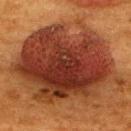No biopsy was performed on this lesion — it was imaged during a full skin examination and was not determined to be concerning.
The lesion's longest dimension is about 13 mm.
Located on the upper back.
The total-body-photography lesion software estimated a footprint of about 85 mm², a shape eccentricity near 0.65, and two-axis asymmetry of about 0.15. The analysis additionally found border irregularity of about 3.5 on a 0–10 scale and a within-lesion color-variation index near 7/10. The analysis additionally found a lesion-detection confidence of about 100/100.
This image is a 15 mm lesion crop taken from a total-body photograph.
The patient is a female roughly 50 years of age.
This is a cross-polarized tile.